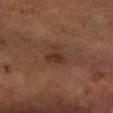| feature | finding |
|---|---|
| workup | catalogued during a skin exam; not biopsied |
| automated lesion analysis | an area of roughly 5 mm², an outline eccentricity of about 0.55 (0 = round, 1 = elongated), and two-axis asymmetry of about 0.55; a mean CIELAB color near L≈32 a*≈19 b*≈25, about 7 CIELAB-L* units darker than the surrounding skin, and a normalized lesion–skin contrast near 6.5; a border-irregularity rating of about 5/10, internal color variation of about 1.5 on a 0–10 scale, and radial color variation of about 0.5 |
| tile lighting | cross-polarized illumination |
| site | the arm |
| patient | male, aged 53 to 57 |
| image | ~15 mm tile from a whole-body skin photo |